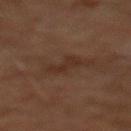The lesion was tiled from a total-body skin photograph and was not biopsied. The subject is a male aged around 60. Located on the chest. The tile uses cross-polarized illumination. About 3 mm across. A 15 mm crop from a total-body photograph taken for skin-cancer surveillance.